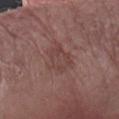The lesion was photographed on a routine skin check and not biopsied; there is no pathology result. The lesion's longest dimension is about 4.5 mm. The lesion is located on the left forearm. The lesion-visualizer software estimated a footprint of about 9.5 mm², an eccentricity of roughly 0.75, and two-axis asymmetry of about 0.3. The software also gave an average lesion color of about L≈42 a*≈20 b*≈21 (CIELAB), a lesion–skin lightness drop of about 6, and a lesion-to-skin contrast of about 5 (normalized; higher = more distinct). The patient is a female aged 83 or older. This image is a 15 mm lesion crop taken from a total-body photograph.